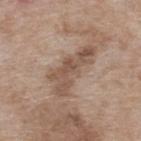notes = catalogued during a skin exam; not biopsied
location = the upper back
image = ~15 mm crop, total-body skin-cancer survey
subject = female, aged around 75
lighting = white-light
diameter = ≈6.5 mm